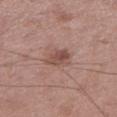notes = imaged on a skin check; not biopsied
image = total-body-photography crop, ~15 mm field of view
size = about 3.5 mm
subject = male, aged around 50
lighting = white-light illumination
site = the left lower leg